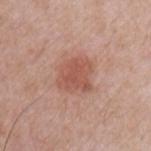Clinical impression: No biopsy was performed on this lesion — it was imaged during a full skin examination and was not determined to be concerning. Image and clinical context: A male patient aged 48 to 52. Captured under white-light illumination. The lesion-visualizer software estimated a footprint of about 11 mm², an eccentricity of roughly 0.4, and a symmetry-axis asymmetry near 0.2. A 15 mm crop from a total-body photograph taken for skin-cancer surveillance. Located on the front of the torso.